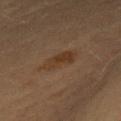biopsy_status: not biopsied; imaged during a skin examination
patient:
  sex: female
  age_approx: 60
lesion_size:
  long_diameter_mm_approx: 4.5
image:
  source: total-body photography crop
  field_of_view_mm: 15
site: mid back
lighting: cross-polarized